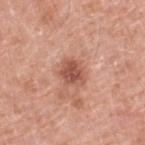{
  "biopsy_status": "not biopsied; imaged during a skin examination",
  "image": {
    "source": "total-body photography crop",
    "field_of_view_mm": 15
  },
  "site": "right lower leg",
  "automated_metrics": {
    "cielab_L": 54,
    "cielab_a": 26,
    "cielab_b": 29,
    "vs_skin_contrast_norm": 8.0,
    "border_irregularity_0_10": 2.0,
    "color_variation_0_10": 3.0,
    "peripheral_color_asymmetry": 1.0
  },
  "lighting": "white-light",
  "patient": {
    "sex": "male",
    "age_approx": 80
  },
  "lesion_size": {
    "long_diameter_mm_approx": 3.5
  }
}